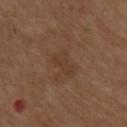{"biopsy_status": "not biopsied; imaged during a skin examination", "image": {"source": "total-body photography crop", "field_of_view_mm": 15}, "patient": {"sex": "male", "age_approx": 75}, "site": "right upper arm", "lighting": "white-light", "lesion_size": {"long_diameter_mm_approx": 3.5}}